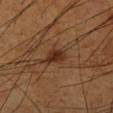Assessment: The lesion was tiled from a total-body skin photograph and was not biopsied. Context: Imaged with cross-polarized lighting. The lesion-visualizer software estimated a lesion color around L≈30 a*≈20 b*≈30 in CIELAB. A male subject, roughly 60 years of age. A region of skin cropped from a whole-body photographic capture, roughly 15 mm wide. Located on the arm.